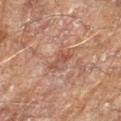Image and clinical context:
The lesion is on the left forearm. Cropped from a whole-body photographic skin survey; the tile spans about 15 mm. The recorded lesion diameter is about 2.5 mm. An algorithmic analysis of the crop reported an eccentricity of roughly 0.85 and a symmetry-axis asymmetry near 0.45. It also reported a lesion color around L≈38 a*≈18 b*≈23 in CIELAB and a normalized border contrast of about 6. And it measured border irregularity of about 5.5 on a 0–10 scale and internal color variation of about 0.5 on a 0–10 scale. It also reported a classifier nevus-likeness of about 0/100 and a detector confidence of about 95 out of 100 that the crop contains a lesion. A male subject, roughly 60 years of age.